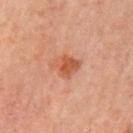Findings:
• subject · male, in their mid- to late 60s
• acquisition · ~15 mm tile from a whole-body skin photo
• body site · the left upper arm
• tile lighting · cross-polarized